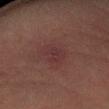Captured during whole-body skin photography for melanoma surveillance; the lesion was not biopsied.
Measured at roughly 3 mm in maximum diameter.
Automated tile analysis of the lesion measured a lesion area of about 5.5 mm² and a shape eccentricity near 0.65. The software also gave a lesion color around L≈28 a*≈19 b*≈16 in CIELAB and a normalized border contrast of about 5.5. And it measured a nevus-likeness score of about 0/100 and lesion-presence confidence of about 100/100.
The tile uses cross-polarized illumination.
A 15 mm close-up extracted from a 3D total-body photography capture.
From the left arm.
A male subject aged approximately 55.